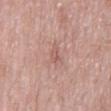{
  "biopsy_status": "not biopsied; imaged during a skin examination",
  "site": "mid back",
  "patient": {
    "sex": "male",
    "age_approx": 55
  },
  "lighting": "white-light",
  "image": {
    "source": "total-body photography crop",
    "field_of_view_mm": 15
  },
  "lesion_size": {
    "long_diameter_mm_approx": 2.5
  },
  "automated_metrics": {
    "eccentricity": 0.85,
    "shape_asymmetry": 0.4,
    "border_irregularity_0_10": 4.0,
    "color_variation_0_10": 1.5,
    "peripheral_color_asymmetry": 0.5
  }
}